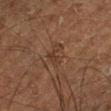Image and clinical context:
From the leg. The tile uses cross-polarized illumination. A region of skin cropped from a whole-body photographic capture, roughly 15 mm wide. Approximately 3 mm at its widest. A male patient, aged approximately 75.